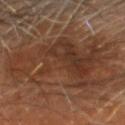Captured during whole-body skin photography for melanoma surveillance; the lesion was not biopsied.
About 11.5 mm across.
This is a cross-polarized tile.
A male patient aged 58 to 62.
Located on the head or neck.
Cropped from a total-body skin-imaging series; the visible field is about 15 mm.